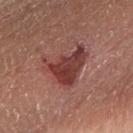Impression: The lesion was tiled from a total-body skin photograph and was not biopsied. Clinical summary: The lesion is on the right forearm. The lesion-visualizer software estimated an area of roughly 16 mm² and an eccentricity of roughly 0.65. It also reported a lesion color around L≈40 a*≈26 b*≈23 in CIELAB and a lesion–skin lightness drop of about 11. It also reported border irregularity of about 6.5 on a 0–10 scale and a within-lesion color-variation index near 5/10. It also reported an automated nevus-likeness rating near 60 out of 100 and lesion-presence confidence of about 100/100. About 5.5 mm across. A 15 mm crop from a total-body photograph taken for skin-cancer surveillance. A female patient, in their 60s.